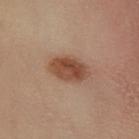Findings:
* biopsy status · imaged on a skin check; not biopsied
* acquisition · ~15 mm tile from a whole-body skin photo
* diameter · about 5 mm
* location · the leg
* subject · female, aged 48 to 52
* TBP lesion metrics · an average lesion color of about L≈39 a*≈18 b*≈26 (CIELAB), about 11 CIELAB-L* units darker than the surrounding skin, and a lesion-to-skin contrast of about 9 (normalized; higher = more distinct); a border-irregularity index near 2/10, internal color variation of about 4 on a 0–10 scale, and a peripheral color-asymmetry measure near 1.5
* tile lighting · cross-polarized